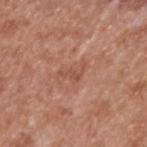notes: total-body-photography surveillance lesion; no biopsy | image source: total-body-photography crop, ~15 mm field of view | lesion diameter: about 3 mm | site: the upper back | TBP lesion metrics: an outline eccentricity of about 0.85 (0 = round, 1 = elongated) and a symmetry-axis asymmetry near 0.75; a mean CIELAB color near L≈51 a*≈24 b*≈30; a nevus-likeness score of about 0/100 and lesion-presence confidence of about 100/100 | subject: male, approximately 65 years of age | illumination: white-light.